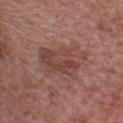No biopsy was performed on this lesion — it was imaged during a full skin examination and was not determined to be concerning. A female subject aged around 55. On the head or neck. Automated image analysis of the tile measured a mean CIELAB color near L≈44 a*≈22 b*≈24, a lesion–skin lightness drop of about 8, and a normalized lesion–skin contrast near 6. The tile uses white-light illumination. A 15 mm close-up tile from a total-body photography series done for melanoma screening. Measured at roughly 6 mm in maximum diameter.